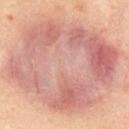notes: imaged on a skin check; not biopsied | image source: 15 mm crop, total-body photography | location: the upper back | subject: male, aged 33–37 | lesion size: ≈13 mm | TBP lesion metrics: a lesion area of about 120 mm² and a shape-asymmetry score of about 0.15 (0 = symmetric); a lesion color around L≈63 a*≈22 b*≈25 in CIELAB; a border-irregularity rating of about 2/10, internal color variation of about 7 on a 0–10 scale, and a peripheral color-asymmetry measure near 2; an automated nevus-likeness rating near 10 out of 100 | lighting: cross-polarized illumination.